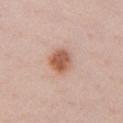<tbp_lesion>
<biopsy_status>not biopsied; imaged during a skin examination</biopsy_status>
<lighting>white-light</lighting>
<automated_metrics>
  <vs_skin_darker_L>13.0</vs_skin_darker_L>
  <vs_skin_contrast_norm>9.0</vs_skin_contrast_norm>
  <nevus_likeness_0_100>100</nevus_likeness_0_100>
  <lesion_detection_confidence_0_100>100</lesion_detection_confidence_0_100>
</automated_metrics>
<image>
  <source>total-body photography crop</source>
  <field_of_view_mm>15</field_of_view_mm>
</image>
<lesion_size>
  <long_diameter_mm_approx>3.0</long_diameter_mm_approx>
</lesion_size>
<patient>
  <sex>female</sex>
  <age_approx>25</age_approx>
</patient>
<site>chest</site>
</tbp_lesion>